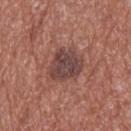Imaged during a routine full-body skin examination; the lesion was not biopsied and no histopathology is available. A male subject aged 73–77. On the upper back. Cropped from a total-body skin-imaging series; the visible field is about 15 mm.